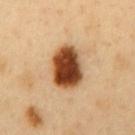Impression: No biopsy was performed on this lesion — it was imaged during a full skin examination and was not determined to be concerning. Context: The subject is a male aged approximately 50. From the mid back. The tile uses cross-polarized illumination. Cropped from a total-body skin-imaging series; the visible field is about 15 mm.